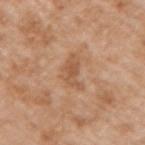This lesion was catalogued during total-body skin photography and was not selected for biopsy. The tile uses white-light illumination. A 15 mm crop from a total-body photograph taken for skin-cancer surveillance. From the right upper arm. A male patient aged 63–67.